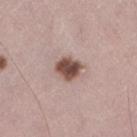diameter — ~3.5 mm (longest diameter) | automated lesion analysis — an eccentricity of roughly 0.65 and a shape-asymmetry score of about 0.2 (0 = symmetric); a lesion color around L≈49 a*≈19 b*≈23 in CIELAB, about 18 CIELAB-L* units darker than the surrounding skin, and a normalized lesion–skin contrast near 12; a border-irregularity index near 2/10, internal color variation of about 4.5 on a 0–10 scale, and radial color variation of about 1.5 | anatomic site — the right thigh | subject — male, in their mid- to late 40s | image source — 15 mm crop, total-body photography.